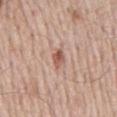Imaged during a routine full-body skin examination; the lesion was not biopsied and no histopathology is available. From the mid back. Automated tile analysis of the lesion measured a lesion color around L≈56 a*≈22 b*≈28 in CIELAB, roughly 12 lightness units darker than nearby skin, and a normalized border contrast of about 8. It also reported a nevus-likeness score of about 90/100 and a detector confidence of about 100 out of 100 that the crop contains a lesion. Measured at roughly 2.5 mm in maximum diameter. The subject is a male aged 63 to 67. A 15 mm close-up extracted from a 3D total-body photography capture.